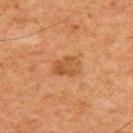follow-up = imaged on a skin check; not biopsied
imaging modality = 15 mm crop, total-body photography
lighting = cross-polarized
patient = male, aged 58 to 62
location = the chest
size = about 3 mm